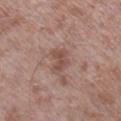Q: Is there a histopathology result?
A: no biopsy performed (imaged during a skin exam)
Q: What lighting was used for the tile?
A: white-light illumination
Q: Automated lesion metrics?
A: a footprint of about 5 mm², an outline eccentricity of about 0.8 (0 = round, 1 = elongated), and two-axis asymmetry of about 0.35; border irregularity of about 4 on a 0–10 scale, internal color variation of about 2.5 on a 0–10 scale, and a peripheral color-asymmetry measure near 1; a detector confidence of about 100 out of 100 that the crop contains a lesion
Q: Who is the patient?
A: male, approximately 55 years of age
Q: How was this image acquired?
A: total-body-photography crop, ~15 mm field of view
Q: What is the lesion's diameter?
A: about 3 mm
Q: Where on the body is the lesion?
A: the right lower leg